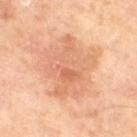Background:
Cropped from a total-body skin-imaging series; the visible field is about 15 mm. A male subject, about 65 years old. Automated image analysis of the tile measured a shape-asymmetry score of about 0.3 (0 = symmetric). It also reported a within-lesion color-variation index near 5/10 and a peripheral color-asymmetry measure near 1.5. Measured at roughly 7.5 mm in maximum diameter. Captured under cross-polarized illumination.